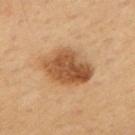Recorded during total-body skin imaging; not selected for excision or biopsy.
On the mid back.
A lesion tile, about 15 mm wide, cut from a 3D total-body photograph.
The subject is a male aged around 65.